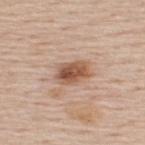Clinical impression:
The lesion was tiled from a total-body skin photograph and was not biopsied.
Background:
The lesion's longest dimension is about 3.5 mm. The lesion is on the upper back. Cropped from a total-body skin-imaging series; the visible field is about 15 mm. Automated image analysis of the tile measured an area of roughly 7.5 mm² and a symmetry-axis asymmetry near 0.2. And it measured border irregularity of about 2 on a 0–10 scale and peripheral color asymmetry of about 2. The tile uses white-light illumination. A male subject aged 58 to 62.